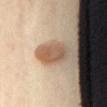Q: Is there a histopathology result?
A: imaged on a skin check; not biopsied
Q: Where on the body is the lesion?
A: the chest
Q: Who is the patient?
A: female, aged 38–42
Q: What kind of image is this?
A: total-body-photography crop, ~15 mm field of view
Q: How large is the lesion?
A: about 4 mm
Q: What did automated image analysis measure?
A: a lesion area of about 8.5 mm² and a symmetry-axis asymmetry near 0.2; a border-irregularity index near 2/10, a color-variation rating of about 4.5/10, and a peripheral color-asymmetry measure near 1.5
Q: Illumination type?
A: cross-polarized